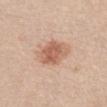No biopsy was performed on this lesion — it was imaged during a full skin examination and was not determined to be concerning. A region of skin cropped from a whole-body photographic capture, roughly 15 mm wide. Automated image analysis of the tile measured a border-irregularity rating of about 1.5/10 and a peripheral color-asymmetry measure near 1. Located on the chest. A female patient, approximately 70 years of age.